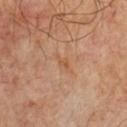biopsy status=no biopsy performed (imaged during a skin exam)
imaging modality=~15 mm tile from a whole-body skin photo
patient=male, aged 53 to 57
anatomic site=the front of the torso